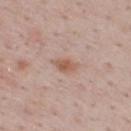The lesion is located on the mid back. The patient is a male aged 48 to 52. A 15 mm crop from a total-body photograph taken for skin-cancer surveillance.